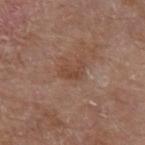Case summary:
- workup: total-body-photography surveillance lesion; no biopsy
- tile lighting: white-light
- patient: male, roughly 80 years of age
- anatomic site: the left upper arm
- image source: total-body-photography crop, ~15 mm field of view